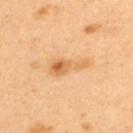notes: catalogued during a skin exam; not biopsied | image: 15 mm crop, total-body photography | subject: male, aged around 40 | body site: the upper back.